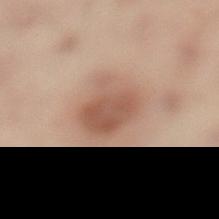diameter — ~4.5 mm (longest diameter) | subject — male, aged 48–52 | location — the leg | imaging modality — ~15 mm crop, total-body skin-cancer survey | tile lighting — cross-polarized illumination.